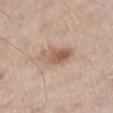{
  "site": "right lower leg",
  "lesion_size": {
    "long_diameter_mm_approx": 4.5
  },
  "automated_metrics": {
    "area_mm2_approx": 9.5,
    "eccentricity": 0.85,
    "shape_asymmetry": 0.2,
    "border_irregularity_0_10": 2.5,
    "color_variation_0_10": 7.0,
    "peripheral_color_asymmetry": 2.5
  },
  "patient": {
    "sex": "male",
    "age_approx": 60
  },
  "image": {
    "source": "total-body photography crop",
    "field_of_view_mm": 15
  },
  "lighting": "white-light"
}